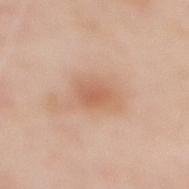<case>
<biopsy_status>not biopsied; imaged during a skin examination</biopsy_status>
<lesion_size>
  <long_diameter_mm_approx>3.0</long_diameter_mm_approx>
</lesion_size>
<patient>
  <sex>female</sex>
  <age_approx>50</age_approx>
</patient>
<automated_metrics>
  <area_mm2_approx>5.5</area_mm2_approx>
  <eccentricity>0.75</eccentricity>
  <shape_asymmetry>0.25</shape_asymmetry>
  <cielab_L>61</cielab_L>
  <cielab_a>23</cielab_a>
  <cielab_b>33</cielab_b>
  <vs_skin_darker_L>8.0</vs_skin_darker_L>
  <border_irregularity_0_10>2.0</border_irregularity_0_10>
  <color_variation_0_10>2.0</color_variation_0_10>
  <peripheral_color_asymmetry>0.5</peripheral_color_asymmetry>
  <nevus_likeness_0_100>20</nevus_likeness_0_100>
</automated_metrics>
<image>
  <source>total-body photography crop</source>
  <field_of_view_mm>15</field_of_view_mm>
</image>
<lighting>white-light</lighting>
<site>back</site>
</case>